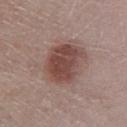workup = catalogued during a skin exam; not biopsied | lesion size = ≈5 mm | patient = male, aged 63–67 | acquisition = ~15 mm tile from a whole-body skin photo | location = the leg.